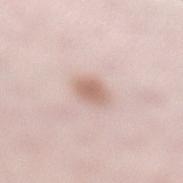Part of a total-body skin-imaging series; this lesion was reviewed on a skin check and was not flagged for biopsy.
A 15 mm close-up tile from a total-body photography series done for melanoma screening.
Located on the left lower leg.
A female patient about 30 years old.